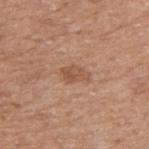A male subject, aged around 65. Cropped from a total-body skin-imaging series; the visible field is about 15 mm. The lesion is on the right upper arm. An algorithmic analysis of the crop reported a lesion area of about 4.5 mm² and an eccentricity of roughly 0.85. The software also gave a border-irregularity rating of about 3/10, a within-lesion color-variation index near 3/10, and peripheral color asymmetry of about 1. Imaged with white-light lighting.